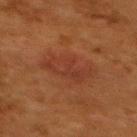Assessment: The lesion was photographed on a routine skin check and not biopsied; there is no pathology result. Background: From the upper back. A female subject aged approximately 50. A roughly 15 mm field-of-view crop from a total-body skin photograph. The lesion's longest dimension is about 5 mm. This is a cross-polarized tile.